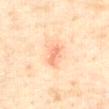Impression: This lesion was catalogued during total-body skin photography and was not selected for biopsy. Background: A male subject approximately 70 years of age. The lesion is located on the abdomen. A close-up tile cropped from a whole-body skin photograph, about 15 mm across.